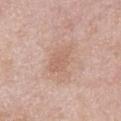A male subject aged around 55. The lesion is on the front of the torso. Longest diameter approximately 4.5 mm. A 15 mm crop from a total-body photograph taken for skin-cancer surveillance. Captured under white-light illumination.